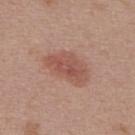Assessment:
Imaged during a routine full-body skin examination; the lesion was not biopsied and no histopathology is available.
Clinical summary:
Captured under white-light illumination. A female patient aged around 45. Cropped from a total-body skin-imaging series; the visible field is about 15 mm. An algorithmic analysis of the crop reported a symmetry-axis asymmetry near 0.25. The lesion's longest dimension is about 5.5 mm. The lesion is located on the back.